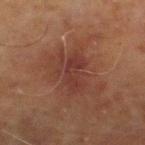notes = total-body-photography surveillance lesion; no biopsy | automated lesion analysis = a border-irregularity rating of about 7/10, a within-lesion color-variation index near 2/10, and radial color variation of about 0.5 | subject = male, aged 68 to 72 | acquisition = 15 mm crop, total-body photography | anatomic site = the right lower leg | diameter = about 4.5 mm.